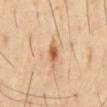workup: no biopsy performed (imaged during a skin exam) | illumination: cross-polarized | TBP lesion metrics: an outline eccentricity of about 0.8 (0 = round, 1 = elongated) and two-axis asymmetry of about 0.2; an average lesion color of about L≈58 a*≈21 b*≈38 (CIELAB) and roughly 12 lightness units darker than nearby skin; border irregularity of about 2 on a 0–10 scale, internal color variation of about 4 on a 0–10 scale, and peripheral color asymmetry of about 1 | subject: male, aged around 55 | image source: total-body-photography crop, ~15 mm field of view | anatomic site: the abdomen | lesion size: ~2.5 mm (longest diameter).